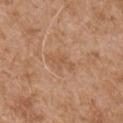| feature | finding |
|---|---|
| workup | imaged on a skin check; not biopsied |
| acquisition | total-body-photography crop, ~15 mm field of view |
| site | the right upper arm |
| patient | male, in their mid-70s |